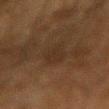No biopsy was performed on this lesion — it was imaged during a full skin examination and was not determined to be concerning.
From the arm.
Automated image analysis of the tile measured an area of roughly 7 mm², an outline eccentricity of about 0.9 (0 = round, 1 = elongated), and a shape-asymmetry score of about 0.25 (0 = symmetric). The software also gave border irregularity of about 3 on a 0–10 scale, a color-variation rating of about 1.5/10, and peripheral color asymmetry of about 0.5.
A male patient aged approximately 60.
A 15 mm crop from a total-body photograph taken for skin-cancer surveillance.